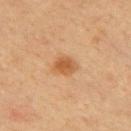The lesion was photographed on a routine skin check and not biopsied; there is no pathology result. The lesion is located on the upper back. A male patient approximately 40 years of age. This is a cross-polarized tile. Cropped from a total-body skin-imaging series; the visible field is about 15 mm. About 3 mm across. The total-body-photography lesion software estimated a mean CIELAB color near L≈50 a*≈21 b*≈37, about 10 CIELAB-L* units darker than the surrounding skin, and a lesion-to-skin contrast of about 8 (normalized; higher = more distinct). It also reported an automated nevus-likeness rating near 95 out of 100.